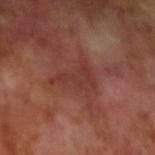workup = catalogued during a skin exam; not biopsied
image = total-body-photography crop, ~15 mm field of view
subject = male, about 70 years old
lesion diameter = ~4.5 mm (longest diameter)
site = the arm
tile lighting = cross-polarized illumination